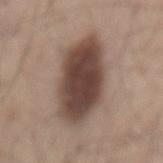Findings:
* biopsy status — imaged on a skin check; not biopsied
* image-analysis metrics — an area of roughly 30 mm², an outline eccentricity of about 0.8 (0 = round, 1 = elongated), and a shape-asymmetry score of about 0.15 (0 = symmetric); a border-irregularity index near 2/10, a within-lesion color-variation index near 5/10, and a peripheral color-asymmetry measure near 1.5
* illumination — white-light
* patient — male, aged around 55
* size — ≈8 mm
* imaging modality — ~15 mm crop, total-body skin-cancer survey
* anatomic site — the mid back